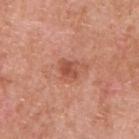  biopsy_status: not biopsied; imaged during a skin examination
  site: upper back
  lighting: white-light
  patient:
    sex: male
    age_approx: 60
  lesion_size:
    long_diameter_mm_approx: 2.5
  automated_metrics:
    color_variation_0_10: 2.5
    peripheral_color_asymmetry: 1.0
  image:
    source: total-body photography crop
    field_of_view_mm: 15